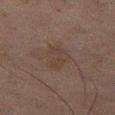{"biopsy_status": "not biopsied; imaged during a skin examination", "patient": {"sex": "male", "age_approx": 60}, "automated_metrics": {"area_mm2_approx": 6.5, "eccentricity": 0.75, "shape_asymmetry": 0.3, "cielab_L": 28, "cielab_a": 11, "cielab_b": 18, "vs_skin_darker_L": 3.0, "vs_skin_contrast_norm": 4.5, "nevus_likeness_0_100": 5}, "lesion_size": {"long_diameter_mm_approx": 3.5}, "image": {"source": "total-body photography crop", "field_of_view_mm": 15}, "site": "left thigh", "lighting": "cross-polarized"}